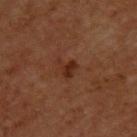notes: catalogued during a skin exam; not biopsied
automated metrics: an area of roughly 3.5 mm² and a shape-asymmetry score of about 0.45 (0 = symmetric); a lesion–skin lightness drop of about 7 and a normalized border contrast of about 7.5; border irregularity of about 4.5 on a 0–10 scale
imaging modality: total-body-photography crop, ~15 mm field of view
location: the upper back
subject: male, aged approximately 60
diameter: ~2.5 mm (longest diameter)